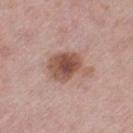A close-up tile cropped from a whole-body skin photograph, about 15 mm across. Approximately 5 mm at its widest. The lesion is located on the left thigh. The subject is a female roughly 40 years of age. Automated tile analysis of the lesion measured a lesion area of about 14 mm² and an eccentricity of roughly 0.65. The software also gave a lesion color around L≈53 a*≈21 b*≈26 in CIELAB, about 13 CIELAB-L* units darker than the surrounding skin, and a normalized lesion–skin contrast near 9. Imaged with white-light lighting.